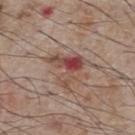<case>
  <biopsy_status>not biopsied; imaged during a skin examination</biopsy_status>
  <automated_metrics>
    <area_mm2_approx>10.0</area_mm2_approx>
    <eccentricity>0.3</eccentricity>
    <shape_asymmetry>0.6</shape_asymmetry>
    <cielab_L>48</cielab_L>
    <cielab_a>22</cielab_a>
    <cielab_b>24</cielab_b>
    <vs_skin_darker_L>10.0</vs_skin_darker_L>
    <vs_skin_contrast_norm>7.5</vs_skin_contrast_norm>
    <border_irregularity_0_10>7.0</border_irregularity_0_10>
    <color_variation_0_10>10.0</color_variation_0_10>
    <peripheral_color_asymmetry>5.5</peripheral_color_asymmetry>
  </automated_metrics>
  <patient>
    <sex>male</sex>
    <age_approx>75</age_approx>
  </patient>
  <image>
    <source>total-body photography crop</source>
    <field_of_view_mm>15</field_of_view_mm>
  </image>
  <site>chest</site>
  <lighting>white-light</lighting>
</case>